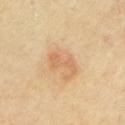- biopsy status — imaged on a skin check; not biopsied
- tile lighting — cross-polarized
- location — the left upper arm
- patient — male, in their mid- to late 60s
- image source — total-body-photography crop, ~15 mm field of view
- lesion diameter — about 3.5 mm